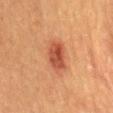About 4.5 mm across.
The lesion is on the mid back.
This is a cross-polarized tile.
A 15 mm close-up tile from a total-body photography series done for melanoma screening.
A male subject aged 48–52.